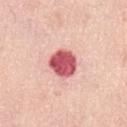Impression: Part of a total-body skin-imaging series; this lesion was reviewed on a skin check and was not flagged for biopsy.